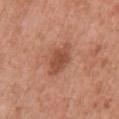{"biopsy_status": "not biopsied; imaged during a skin examination", "patient": {"sex": "female", "age_approx": 50}, "image": {"source": "total-body photography crop", "field_of_view_mm": 15}, "site": "chest", "lesion_size": {"long_diameter_mm_approx": 4.5}}